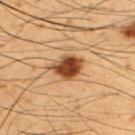Impression:
No biopsy was performed on this lesion — it was imaged during a full skin examination and was not determined to be concerning.
Acquisition and patient details:
A region of skin cropped from a whole-body photographic capture, roughly 15 mm wide. Located on the upper back. A male patient, aged 48–52.